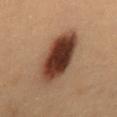Recorded during total-body skin imaging; not selected for excision or biopsy.
On the mid back.
The subject is a male approximately 55 years of age.
A 15 mm close-up extracted from a 3D total-body photography capture.
An algorithmic analysis of the crop reported an area of roughly 22 mm², an outline eccentricity of about 0.8 (0 = round, 1 = elongated), and a shape-asymmetry score of about 0.1 (0 = symmetric). And it measured a border-irregularity rating of about 1.5/10, a color-variation rating of about 7/10, and radial color variation of about 2.
Longest diameter approximately 7 mm.
This is a cross-polarized tile.